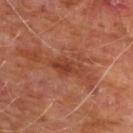Q: Is there a histopathology result?
A: no biopsy performed (imaged during a skin exam)
Q: What did automated image analysis measure?
A: a mean CIELAB color near L≈39 a*≈26 b*≈31, roughly 8 lightness units darker than nearby skin, and a normalized border contrast of about 6.5; border irregularity of about 2.5 on a 0–10 scale and peripheral color asymmetry of about 1.5
Q: What kind of image is this?
A: total-body-photography crop, ~15 mm field of view
Q: Who is the patient?
A: male, approximately 60 years of age
Q: Lesion location?
A: the chest
Q: Illumination type?
A: cross-polarized
Q: Lesion size?
A: ~4 mm (longest diameter)